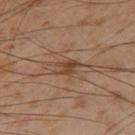<case>
  <biopsy_status>not biopsied; imaged during a skin examination</biopsy_status>
</case>